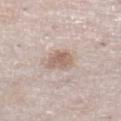{"biopsy_status": "not biopsied; imaged during a skin examination", "image": {"source": "total-body photography crop", "field_of_view_mm": 15}, "site": "left lower leg", "patient": {"sex": "female", "age_approx": 30}, "lesion_size": {"long_diameter_mm_approx": 3.5}, "lighting": "white-light"}